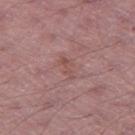Findings:
• follow-up — no biopsy performed (imaged during a skin exam)
• imaging modality — 15 mm crop, total-body photography
• diameter — about 3 mm
• TBP lesion metrics — an area of roughly 3 mm² and an eccentricity of roughly 0.9
• anatomic site — the leg
• patient — male, aged 63–67
• illumination — white-light illumination